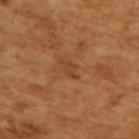Case summary:
- follow-up — imaged on a skin check; not biopsied
- image — ~15 mm tile from a whole-body skin photo
- image-analysis metrics — a lesion area of about 3 mm² and two-axis asymmetry of about 0.3; a mean CIELAB color near L≈41 a*≈21 b*≈35 and a lesion-to-skin contrast of about 5.5 (normalized; higher = more distinct); a within-lesion color-variation index near 1/10 and a peripheral color-asymmetry measure near 0.5; a classifier nevus-likeness of about 0/100
- lesion size — ~2.5 mm (longest diameter)
- lighting — cross-polarized
- patient — male, about 65 years old